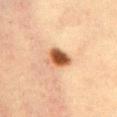The subject is a female approximately 40 years of age. Measured at roughly 3.5 mm in maximum diameter. Cropped from a total-body skin-imaging series; the visible field is about 15 mm. Imaged with cross-polarized lighting. On the front of the torso.